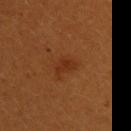Assessment:
The lesion was tiled from a total-body skin photograph and was not biopsied.
Acquisition and patient details:
A female patient approximately 30 years of age. Longest diameter approximately 2.5 mm. The total-body-photography lesion software estimated an area of roughly 3 mm², a shape eccentricity near 0.8, and two-axis asymmetry of about 0.45. The software also gave border irregularity of about 4.5 on a 0–10 scale, a color-variation rating of about 1.5/10, and radial color variation of about 0.5. The analysis additionally found a nevus-likeness score of about 35/100. A 15 mm close-up tile from a total-body photography series done for melanoma screening. Imaged with cross-polarized lighting. On the left upper arm.